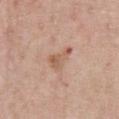notes: total-body-photography surveillance lesion; no biopsy
illumination: white-light illumination
automated lesion analysis: a lesion area of about 5 mm² and two-axis asymmetry of about 0.6; an average lesion color of about L≈58 a*≈21 b*≈30 (CIELAB) and a normalized lesion–skin contrast near 6.5
acquisition: ~15 mm tile from a whole-body skin photo
lesion diameter: ≈3.5 mm
site: the chest
subject: male, about 60 years old